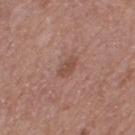<tbp_lesion>
<biopsy_status>not biopsied; imaged during a skin examination</biopsy_status>
<image>
  <source>total-body photography crop</source>
  <field_of_view_mm>15</field_of_view_mm>
</image>
<lighting>white-light</lighting>
<patient>
  <sex>female</sex>
  <age_approx>40</age_approx>
</patient>
<lesion_size>
  <long_diameter_mm_approx>2.5</long_diameter_mm_approx>
</lesion_size>
<site>leg</site>
</tbp_lesion>